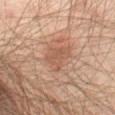{
  "biopsy_status": "not biopsied; imaged during a skin examination",
  "image": {
    "source": "total-body photography crop",
    "field_of_view_mm": 15
  },
  "patient": {
    "sex": "male",
    "age_approx": 65
  },
  "lighting": "cross-polarized",
  "site": "abdomen",
  "lesion_size": {
    "long_diameter_mm_approx": 3.5
  }
}